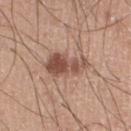Acquisition and patient details:
Cropped from a whole-body photographic skin survey; the tile spans about 15 mm. A male subject, roughly 20 years of age. The lesion is located on the right lower leg. Captured under white-light illumination.